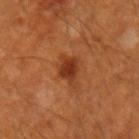Q: Was this lesion biopsied?
A: imaged on a skin check; not biopsied
Q: What is the anatomic site?
A: the right forearm
Q: How was the tile lit?
A: cross-polarized
Q: What kind of image is this?
A: total-body-photography crop, ~15 mm field of view
Q: What are the patient's age and sex?
A: male, aged 58–62
Q: Automated lesion metrics?
A: a lesion color around L≈35 a*≈26 b*≈34 in CIELAB and about 9 CIELAB-L* units darker than the surrounding skin
Q: How large is the lesion?
A: ~4 mm (longest diameter)